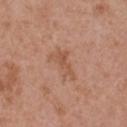This lesion was catalogued during total-body skin photography and was not selected for biopsy.
The lesion's longest dimension is about 4 mm.
Captured under white-light illumination.
Located on the upper back.
Cropped from a whole-body photographic skin survey; the tile spans about 15 mm.
A female patient roughly 40 years of age.